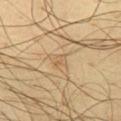The lesion was photographed on a routine skin check and not biopsied; there is no pathology result.
Automated image analysis of the tile measured a footprint of about 2 mm² and a shape eccentricity near 0.7. It also reported a border-irregularity index near 3.5/10 and internal color variation of about 0 on a 0–10 scale. The software also gave a classifier nevus-likeness of about 0/100 and a detector confidence of about 25 out of 100 that the crop contains a lesion.
A 15 mm crop from a total-body photograph taken for skin-cancer surveillance.
Measured at roughly 1.5 mm in maximum diameter.
A male subject roughly 65 years of age.
This is a cross-polarized tile.
The lesion is located on the left thigh.